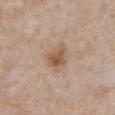Assessment: Recorded during total-body skin imaging; not selected for excision or biopsy. Clinical summary: The tile uses white-light illumination. This image is a 15 mm lesion crop taken from a total-body photograph. The subject is a male aged around 65. The lesion's longest dimension is about 3 mm. Located on the abdomen. An algorithmic analysis of the crop reported an average lesion color of about L≈55 a*≈19 b*≈30 (CIELAB) and a normalized border contrast of about 7.5. It also reported a border-irregularity index near 3/10 and peripheral color asymmetry of about 1.